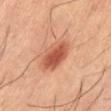Clinical impression: No biopsy was performed on this lesion — it was imaged during a full skin examination and was not determined to be concerning. Image and clinical context: The lesion is located on the abdomen. A region of skin cropped from a whole-body photographic capture, roughly 15 mm wide. A male patient, roughly 40 years of age.